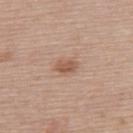No biopsy was performed on this lesion — it was imaged during a full skin examination and was not determined to be concerning. Imaged with white-light lighting. The lesion is on the upper back. Longest diameter approximately 2.5 mm. Cropped from a total-body skin-imaging series; the visible field is about 15 mm. The subject is a female aged 63 to 67.